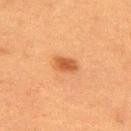Q: Was this lesion biopsied?
A: catalogued during a skin exam; not biopsied
Q: Lesion size?
A: ≈3 mm
Q: What did automated image analysis measure?
A: a lesion area of about 4.5 mm², an outline eccentricity of about 0.75 (0 = round, 1 = elongated), and a symmetry-axis asymmetry near 0.2; a border-irregularity rating of about 2/10
Q: What are the patient's age and sex?
A: female, about 55 years old
Q: What is the anatomic site?
A: the upper back
Q: What kind of image is this?
A: 15 mm crop, total-body photography